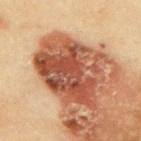No biopsy was performed on this lesion — it was imaged during a full skin examination and was not determined to be concerning.